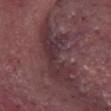workup: catalogued during a skin exam; not biopsied
lesion diameter: ~8.5 mm (longest diameter)
subject: male, aged 83 or over
anatomic site: the head or neck
acquisition: 15 mm crop, total-body photography